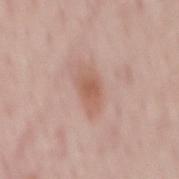Case summary:
- lesion diameter: ~4.5 mm (longest diameter)
- subject: male, approximately 50 years of age
- image source: 15 mm crop, total-body photography
- site: the mid back
- illumination: white-light